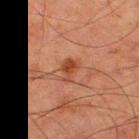Findings:
• workup — catalogued during a skin exam; not biopsied
• patient — male, about 80 years old
• anatomic site — the right thigh
• image — 15 mm crop, total-body photography